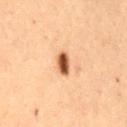Clinical impression: No biopsy was performed on this lesion — it was imaged during a full skin examination and was not determined to be concerning. Acquisition and patient details: A female patient, roughly 80 years of age. Cropped from a whole-body photographic skin survey; the tile spans about 15 mm. The total-body-photography lesion software estimated an area of roughly 4 mm² and a shape eccentricity near 0.8. The analysis additionally found a border-irregularity index near 2/10 and peripheral color asymmetry of about 0.5. And it measured a classifier nevus-likeness of about 100/100 and a lesion-detection confidence of about 100/100. The tile uses cross-polarized illumination. On the mid back. Measured at roughly 3 mm in maximum diameter.